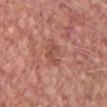Q: What is the imaging modality?
A: ~15 mm tile from a whole-body skin photo
Q: Lesion size?
A: ≈3.5 mm
Q: What lighting was used for the tile?
A: white-light illumination
Q: What are the patient's age and sex?
A: male, aged 48 to 52
Q: Where on the body is the lesion?
A: the chest
Q: What did automated image analysis measure?
A: an average lesion color of about L≈52 a*≈26 b*≈28 (CIELAB), roughly 7 lightness units darker than nearby skin, and a lesion-to-skin contrast of about 5.5 (normalized; higher = more distinct)